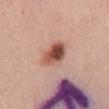On the back. A female patient aged approximately 55. Cropped from a total-body skin-imaging series; the visible field is about 15 mm. Histopathologically confirmed as a dysplastic (Clark) nevus, classified as a benign skin lesion.